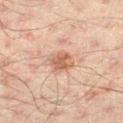| key | value |
|---|---|
| subject | male, roughly 45 years of age |
| location | the right thigh |
| lesion diameter | ~2.5 mm (longest diameter) |
| image source | ~15 mm tile from a whole-body skin photo |